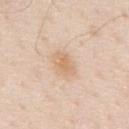Recorded during total-body skin imaging; not selected for excision or biopsy.
The patient is a male roughly 80 years of age.
Measured at roughly 3.5 mm in maximum diameter.
On the mid back.
A region of skin cropped from a whole-body photographic capture, roughly 15 mm wide.